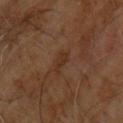The lesion was photographed on a routine skin check and not biopsied; there is no pathology result. A male subject, in their 60s. The lesion-visualizer software estimated a lesion color around L≈29 a*≈18 b*≈28 in CIELAB, a lesion–skin lightness drop of about 6, and a normalized border contrast of about 6.5. It also reported a border-irregularity rating of about 4.5/10, a within-lesion color-variation index near 0/10, and a peripheral color-asymmetry measure near 0. The analysis additionally found a nevus-likeness score of about 0/100 and a detector confidence of about 100 out of 100 that the crop contains a lesion. About 2.5 mm across. A region of skin cropped from a whole-body photographic capture, roughly 15 mm wide. The lesion is located on the front of the torso. Captured under cross-polarized illumination.